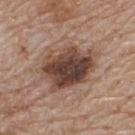This lesion was catalogued during total-body skin photography and was not selected for biopsy. Cropped from a total-body skin-imaging series; the visible field is about 15 mm. A male patient, roughly 80 years of age. From the upper back. Longest diameter approximately 7 mm. The tile uses white-light illumination.